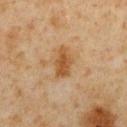The lesion is located on the front of the torso. A roughly 15 mm field-of-view crop from a total-body skin photograph. A male patient, about 60 years old.